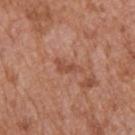<record>
<biopsy_status>not biopsied; imaged during a skin examination</biopsy_status>
<lesion_size>
  <long_diameter_mm_approx>3.5</long_diameter_mm_approx>
</lesion_size>
<patient>
  <sex>male</sex>
  <age_approx>65</age_approx>
</patient>
<lighting>white-light</lighting>
<automated_metrics>
  <area_mm2_approx>3.5</area_mm2_approx>
  <eccentricity>0.9</eccentricity>
  <shape_asymmetry>0.5</shape_asymmetry>
  <border_irregularity_0_10>5.0</border_irregularity_0_10>
  <color_variation_0_10>0.5</color_variation_0_10>
  <nevus_likeness_0_100>0</nevus_likeness_0_100>
  <lesion_detection_confidence_0_100>100</lesion_detection_confidence_0_100>
</automated_metrics>
<site>upper back</site>
<image>
  <source>total-body photography crop</source>
  <field_of_view_mm>15</field_of_view_mm>
</image>
</record>